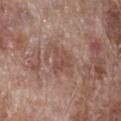No biopsy was performed on this lesion — it was imaged during a full skin examination and was not determined to be concerning.
A male subject, aged 68 to 72.
This is a white-light tile.
Located on the right lower leg.
A region of skin cropped from a whole-body photographic capture, roughly 15 mm wide.
The recorded lesion diameter is about 4.5 mm.